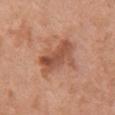This lesion was catalogued during total-body skin photography and was not selected for biopsy. The lesion is located on the chest. This image is a 15 mm lesion crop taken from a total-body photograph. A female subject roughly 65 years of age.